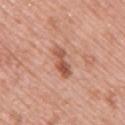<tbp_lesion>
  <biopsy_status>not biopsied; imaged during a skin examination</biopsy_status>
  <patient>
    <sex>male</sex>
    <age_approx>55</age_approx>
  </patient>
  <lesion_size>
    <long_diameter_mm_approx>4.0</long_diameter_mm_approx>
  </lesion_size>
  <image>
    <source>total-body photography crop</source>
    <field_of_view_mm>15</field_of_view_mm>
  </image>
  <site>upper back</site>
  <automated_metrics>
    <cielab_L>55</cielab_L>
    <cielab_a>25</cielab_a>
    <cielab_b>31</cielab_b>
    <vs_skin_contrast_norm>8.0</vs_skin_contrast_norm>
    <nevus_likeness_0_100>55</nevus_likeness_0_100>
    <lesion_detection_confidence_0_100>100</lesion_detection_confidence_0_100>
  </automated_metrics>
</tbp_lesion>